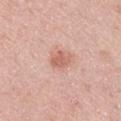Imaged during a routine full-body skin examination; the lesion was not biopsied and no histopathology is available.
A lesion tile, about 15 mm wide, cut from a 3D total-body photograph.
Captured under white-light illumination.
The lesion is located on the left upper arm.
A male subject, about 45 years old.